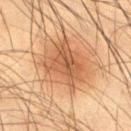Part of a total-body skin-imaging series; this lesion was reviewed on a skin check and was not flagged for biopsy.
Approximately 5.5 mm at its widest.
The lesion is located on the right thigh.
Automated tile analysis of the lesion measured a footprint of about 20 mm², a shape eccentricity near 0.45, and a shape-asymmetry score of about 0.2 (0 = symmetric). And it measured a lesion color around L≈45 a*≈19 b*≈30 in CIELAB, about 10 CIELAB-L* units darker than the surrounding skin, and a normalized lesion–skin contrast near 7.5.
A 15 mm close-up tile from a total-body photography series done for melanoma screening.
A male subject, aged 33 to 37.